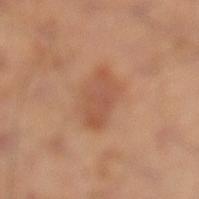Q: Was this lesion biopsied?
A: imaged on a skin check; not biopsied
Q: Who is the patient?
A: male, aged 48 to 52
Q: What did automated image analysis measure?
A: an area of roughly 10 mm², an eccentricity of roughly 0.75, and a shape-asymmetry score of about 0.25 (0 = symmetric); a lesion color around L≈51 a*≈23 b*≈32 in CIELAB, about 8 CIELAB-L* units darker than the surrounding skin, and a lesion-to-skin contrast of about 5.5 (normalized; higher = more distinct)
Q: Where on the body is the lesion?
A: the left leg
Q: What lighting was used for the tile?
A: cross-polarized illumination
Q: Lesion size?
A: ~4.5 mm (longest diameter)
Q: What kind of image is this?
A: ~15 mm crop, total-body skin-cancer survey